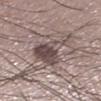On the left lower leg. The total-body-photography lesion software estimated border irregularity of about 6.5 on a 0–10 scale and peripheral color asymmetry of about 2.5. The recorded lesion diameter is about 7 mm. This is a white-light tile. A 15 mm close-up extracted from a 3D total-body photography capture. A male patient in their 50s.